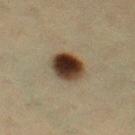Background:
Imaged with cross-polarized lighting. A 15 mm crop from a total-body photograph taken for skin-cancer surveillance. The patient is a female aged around 40. Located on the right thigh.